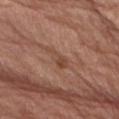Q: What kind of image is this?
A: ~15 mm tile from a whole-body skin photo
Q: Who is the patient?
A: male, aged approximately 70
Q: What lighting was used for the tile?
A: white-light illumination
Q: Lesion size?
A: ≈3.5 mm
Q: Automated lesion metrics?
A: an average lesion color of about L≈46 a*≈21 b*≈29 (CIELAB), a lesion–skin lightness drop of about 7, and a normalized lesion–skin contrast near 6
Q: Where on the body is the lesion?
A: the left thigh